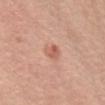The lesion was photographed on a routine skin check and not biopsied; there is no pathology result. A female patient, roughly 45 years of age. From the chest. About 2.5 mm across. An algorithmic analysis of the crop reported a footprint of about 4 mm², an outline eccentricity of about 0.55 (0 = round, 1 = elongated), and a shape-asymmetry score of about 0.25 (0 = symmetric). It also reported an automated nevus-likeness rating near 50 out of 100 and a detector confidence of about 100 out of 100 that the crop contains a lesion. A close-up tile cropped from a whole-body skin photograph, about 15 mm across.